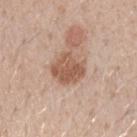Case summary:
* workup: catalogued during a skin exam; not biopsied
* image-analysis metrics: a footprint of about 11 mm² and an outline eccentricity of about 0.35 (0 = round, 1 = elongated)
* acquisition: 15 mm crop, total-body photography
* anatomic site: the arm
* patient: male, about 30 years old
* lesion diameter: ≈4 mm
* lighting: white-light